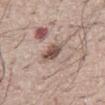- biopsy status: catalogued during a skin exam; not biopsied
- anatomic site: the abdomen
- illumination: white-light illumination
- patient: male, in their mid- to late 60s
- image source: 15 mm crop, total-body photography